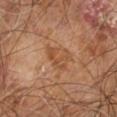Case summary:
– notes · total-body-photography surveillance lesion; no biopsy
– size · ~3.5 mm (longest diameter)
– tile lighting · cross-polarized
– automated metrics · a footprint of about 6.5 mm², an outline eccentricity of about 0.7 (0 = round, 1 = elongated), and a symmetry-axis asymmetry near 0.45
– patient · male, roughly 60 years of age
– image · ~15 mm crop, total-body skin-cancer survey
– location · the right leg